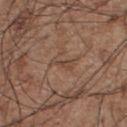Automated tile analysis of the lesion measured a lesion area of about 3.5 mm², an outline eccentricity of about 0.9 (0 = round, 1 = elongated), and a shape-asymmetry score of about 0.35 (0 = symmetric). The analysis additionally found a nevus-likeness score of about 0/100 and lesion-presence confidence of about 60/100.
A male subject, about 55 years old.
Cropped from a whole-body photographic skin survey; the tile spans about 15 mm.
Captured under white-light illumination.
Measured at roughly 3 mm in maximum diameter.
The lesion is on the chest.